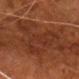A male subject in their 80s. This is a cross-polarized tile. Approximately 12 mm at its widest. A 15 mm close-up extracted from a 3D total-body photography capture. The lesion is on the chest.